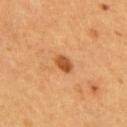notes: imaged on a skin check; not biopsied | image: 15 mm crop, total-body photography | patient: male, aged 53–57 | body site: the upper back.